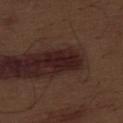Assessment:
No biopsy was performed on this lesion — it was imaged during a full skin examination and was not determined to be concerning.
Clinical summary:
This is a white-light tile. On the abdomen. Approximately 4 mm at its widest. Cropped from a whole-body photographic skin survey; the tile spans about 15 mm. The total-body-photography lesion software estimated roughly 9 lightness units darker than nearby skin and a normalized lesion–skin contrast near 12. A male patient, in their 70s.